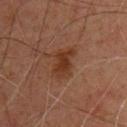Part of a total-body skin-imaging series; this lesion was reviewed on a skin check and was not flagged for biopsy. This is a cross-polarized tile. Cropped from a total-body skin-imaging series; the visible field is about 15 mm. From the chest. Automated tile analysis of the lesion measured a lesion–skin lightness drop of about 7 and a normalized lesion–skin contrast near 8. And it measured border irregularity of about 3.5 on a 0–10 scale and a peripheral color-asymmetry measure near 0.5. A male subject in their mid- to late 40s. Longest diameter approximately 4 mm.